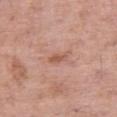Q: What is the anatomic site?
A: the right thigh
Q: What is the lesion's diameter?
A: about 2.5 mm
Q: What are the patient's age and sex?
A: female, aged approximately 60
Q: What kind of image is this?
A: 15 mm crop, total-body photography